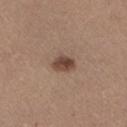notes: no biopsy performed (imaged during a skin exam) | diameter: about 3 mm | site: the right thigh | imaging modality: total-body-photography crop, ~15 mm field of view | tile lighting: white-light illumination | automated lesion analysis: a lesion area of about 5 mm², a shape eccentricity near 0.6, and a shape-asymmetry score of about 0.25 (0 = symmetric); peripheral color asymmetry of about 1.5 | patient: female, aged around 35.